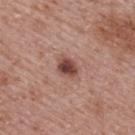Findings:
– follow-up · total-body-photography surveillance lesion; no biopsy
– patient · female, aged approximately 65
– body site · the back
– image-analysis metrics · a mean CIELAB color near L≈46 a*≈23 b*≈24, about 14 CIELAB-L* units darker than the surrounding skin, and a lesion-to-skin contrast of about 10.5 (normalized; higher = more distinct); a classifier nevus-likeness of about 95/100 and a detector confidence of about 100 out of 100 that the crop contains a lesion
– imaging modality · total-body-photography crop, ~15 mm field of view